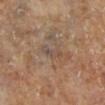{"biopsy_status": "not biopsied; imaged during a skin examination", "patient": {"sex": "female"}, "automated_metrics": {"border_irregularity_0_10": 4.0, "color_variation_0_10": 0.0, "peripheral_color_asymmetry": 0.0}, "site": "left lower leg", "image": {"source": "total-body photography crop", "field_of_view_mm": 15}}